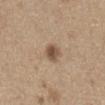Q: Was this lesion biopsied?
A: catalogued during a skin exam; not biopsied
Q: Automated lesion metrics?
A: an area of roughly 5 mm², an outline eccentricity of about 0.55 (0 = round, 1 = elongated), and a shape-asymmetry score of about 0.15 (0 = symmetric); an average lesion color of about L≈52 a*≈16 b*≈28 (CIELAB), roughly 12 lightness units darker than nearby skin, and a normalized border contrast of about 8.5
Q: Lesion size?
A: about 2.5 mm
Q: What is the anatomic site?
A: the abdomen
Q: Illumination type?
A: white-light
Q: Patient demographics?
A: male, approximately 65 years of age
Q: What kind of image is this?
A: ~15 mm crop, total-body skin-cancer survey